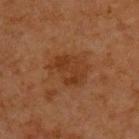This lesion was catalogued during total-body skin photography and was not selected for biopsy.
The subject is a male aged 63–67.
Cropped from a total-body skin-imaging series; the visible field is about 15 mm.
On the upper back.